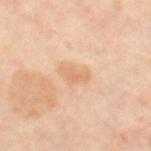notes: imaged on a skin check; not biopsied | automated metrics: an area of roughly 3 mm² and a symmetry-axis asymmetry near 0.4 | image: 15 mm crop, total-body photography | diameter: about 2.5 mm | patient: female, aged approximately 50 | body site: the leg.